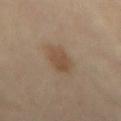Impression: No biopsy was performed on this lesion — it was imaged during a full skin examination and was not determined to be concerning.